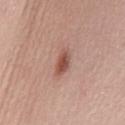Clinical impression: No biopsy was performed on this lesion — it was imaged during a full skin examination and was not determined to be concerning. Context: On the back. Cropped from a total-body skin-imaging series; the visible field is about 15 mm. The subject is a female in their 30s.